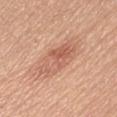Impression: This lesion was catalogued during total-body skin photography and was not selected for biopsy. Acquisition and patient details: A female patient, aged 53–57. The lesion's longest dimension is about 6 mm. The tile uses white-light illumination. A region of skin cropped from a whole-body photographic capture, roughly 15 mm wide. From the abdomen.